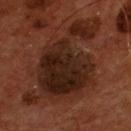Impression:
No biopsy was performed on this lesion — it was imaged during a full skin examination and was not determined to be concerning.
Clinical summary:
Approximately 13 mm at its widest. The subject is a male in their mid-50s. A roughly 15 mm field-of-view crop from a total-body skin photograph. On the chest. An algorithmic analysis of the crop reported a footprint of about 50 mm² and an eccentricity of roughly 0.85. The software also gave a mean CIELAB color near L≈21 a*≈18 b*≈23, roughly 9 lightness units darker than nearby skin, and a normalized border contrast of about 10.5. And it measured a lesion-detection confidence of about 100/100.